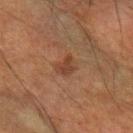<record>
<biopsy_status>not biopsied; imaged during a skin examination</biopsy_status>
<lighting>cross-polarized</lighting>
<patient>
  <sex>male</sex>
  <age_approx>50</age_approx>
</patient>
<image>
  <source>total-body photography crop</source>
  <field_of_view_mm>15</field_of_view_mm>
</image>
<lesion_size>
  <long_diameter_mm_approx>3.0</long_diameter_mm_approx>
</lesion_size>
<site>arm</site>
<automated_metrics>
  <area_mm2_approx>5.0</area_mm2_approx>
  <border_irregularity_0_10>2.5</border_irregularity_0_10>
  <color_variation_0_10>2.5</color_variation_0_10>
</automated_metrics>
</record>